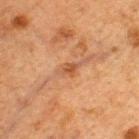biopsy_status: not biopsied; imaged during a skin examination
patient:
  sex: male
  age_approx: 50
site: upper back
image:
  source: total-body photography crop
  field_of_view_mm: 15